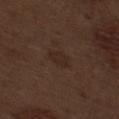The lesion was tiled from a total-body skin photograph and was not biopsied.
On the right thigh.
Approximately 3 mm at its widest.
A 15 mm crop from a total-body photograph taken for skin-cancer surveillance.
The patient is a male aged approximately 70.